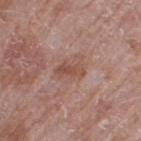Q: Was a biopsy performed?
A: imaged on a skin check; not biopsied
Q: Who is the patient?
A: male, aged 58–62
Q: Where on the body is the lesion?
A: the right thigh
Q: How was this image acquired?
A: 15 mm crop, total-body photography